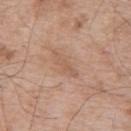Part of a total-body skin-imaging series; this lesion was reviewed on a skin check and was not flagged for biopsy. Measured at roughly 4 mm in maximum diameter. A close-up tile cropped from a whole-body skin photograph, about 15 mm across. Located on the upper back. This is a white-light tile. Automated tile analysis of the lesion measured an area of roughly 4.5 mm², an eccentricity of roughly 0.95, and a symmetry-axis asymmetry near 0.45. The analysis additionally found a lesion color around L≈57 a*≈19 b*≈30 in CIELAB. The analysis additionally found an automated nevus-likeness rating near 0 out of 100 and a lesion-detection confidence of about 100/100. A male patient roughly 65 years of age.